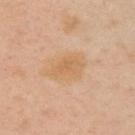Captured during whole-body skin photography for melanoma surveillance; the lesion was not biopsied. The patient is a female in their 40s. Located on the left arm. Longest diameter approximately 4.5 mm. A lesion tile, about 15 mm wide, cut from a 3D total-body photograph.